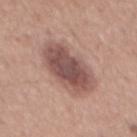workup: no biopsy performed (imaged during a skin exam)
imaging modality: 15 mm crop, total-body photography
lighting: white-light illumination
patient: male, roughly 55 years of age
lesion diameter: ≈7 mm
image-analysis metrics: a border-irregularity rating of about 2.5/10, a color-variation rating of about 5/10, and peripheral color asymmetry of about 1.5; a classifier nevus-likeness of about 35/100 and a detector confidence of about 100 out of 100 that the crop contains a lesion
body site: the mid back